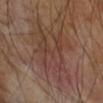Captured during whole-body skin photography for melanoma surveillance; the lesion was not biopsied. A 15 mm crop from a total-body photograph taken for skin-cancer surveillance. The tile uses cross-polarized illumination. Automated image analysis of the tile measured a lesion area of about 31 mm², a shape eccentricity near 0.7, and two-axis asymmetry of about 0.45. The analysis additionally found a lesion–skin lightness drop of about 6. It also reported a nevus-likeness score of about 0/100. About 9 mm across. The lesion is on the right upper arm.